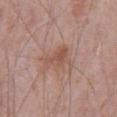<record>
<biopsy_status>not biopsied; imaged during a skin examination</biopsy_status>
<automated_metrics>
  <cielab_L>53</cielab_L>
  <cielab_a>20</cielab_a>
  <cielab_b>27</cielab_b>
  <vs_skin_darker_L>7.0</vs_skin_darker_L>
  <vs_skin_contrast_norm>6.0</vs_skin_contrast_norm>
</automated_metrics>
<lesion_size>
  <long_diameter_mm_approx>4.0</long_diameter_mm_approx>
</lesion_size>
<patient>
  <sex>male</sex>
  <age_approx>70</age_approx>
</patient>
<site>abdomen</site>
<image>
  <source>total-body photography crop</source>
  <field_of_view_mm>15</field_of_view_mm>
</image>
<lighting>white-light</lighting>
</record>